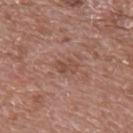Captured during whole-body skin photography for melanoma surveillance; the lesion was not biopsied. Automated tile analysis of the lesion measured an average lesion color of about L≈48 a*≈21 b*≈27 (CIELAB), about 7 CIELAB-L* units darker than the surrounding skin, and a lesion-to-skin contrast of about 5.5 (normalized; higher = more distinct). The software also gave a border-irregularity rating of about 3/10 and internal color variation of about 3 on a 0–10 scale. And it measured a lesion-detection confidence of about 100/100. The tile uses white-light illumination. A roughly 15 mm field-of-view crop from a total-body skin photograph. Approximately 2.5 mm at its widest. A male patient, aged approximately 70. From the upper back.